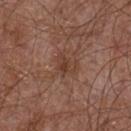<case>
  <biopsy_status>not biopsied; imaged during a skin examination</biopsy_status>
  <automated_metrics>
    <cielab_L>39</cielab_L>
    <cielab_a>20</cielab_a>
    <cielab_b>27</cielab_b>
    <vs_skin_darker_L>7.0</vs_skin_darker_L>
    <vs_skin_contrast_norm>6.5</vs_skin_contrast_norm>
    <lesion_detection_confidence_0_100>100</lesion_detection_confidence_0_100>
  </automated_metrics>
  <patient>
    <sex>male</sex>
    <age_approx>55</age_approx>
  </patient>
  <image>
    <source>total-body photography crop</source>
    <field_of_view_mm>15</field_of_view_mm>
  </image>
  <site>front of the torso</site>
  <lesion_size>
    <long_diameter_mm_approx>2.5</long_diameter_mm_approx>
  </lesion_size>
</case>